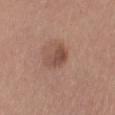{"biopsy_status": "not biopsied; imaged during a skin examination"}